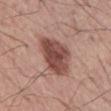biopsy_status: not biopsied; imaged during a skin examination
site: abdomen
patient:
  sex: male
  age_approx: 55
image:
  source: total-body photography crop
  field_of_view_mm: 15